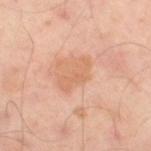Assessment:
The lesion was photographed on a routine skin check and not biopsied; there is no pathology result.
Context:
A male subject, aged around 50. The lesion is located on the left leg. A 15 mm crop from a total-body photograph taken for skin-cancer surveillance. An algorithmic analysis of the crop reported a shape eccentricity near 0.9 and two-axis asymmetry of about 0.45. It also reported a border-irregularity rating of about 6.5/10 and internal color variation of about 1 on a 0–10 scale. The lesion's longest dimension is about 4 mm.